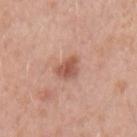biopsy status: total-body-photography surveillance lesion; no biopsy | imaging modality: 15 mm crop, total-body photography | tile lighting: white-light | image-analysis metrics: a footprint of about 5 mm², an outline eccentricity of about 0.55 (0 = round, 1 = elongated), and a shape-asymmetry score of about 0.3 (0 = symmetric); a mean CIELAB color near L≈55 a*≈24 b*≈29, a lesion–skin lightness drop of about 11, and a normalized border contrast of about 7.5; internal color variation of about 2.5 on a 0–10 scale and radial color variation of about 1 | subject: male, approximately 50 years of age | anatomic site: the left upper arm | lesion diameter: ≈3 mm.